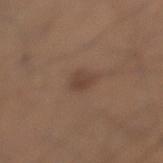Assessment:
This lesion was catalogued during total-body skin photography and was not selected for biopsy.
Image and clinical context:
A male patient, aged 28–32. Located on the left lower leg. A roughly 15 mm field-of-view crop from a total-body skin photograph.